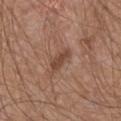workup: imaged on a skin check; not biopsied | automated metrics: a lesion color around L≈45 a*≈20 b*≈28 in CIELAB and about 9 CIELAB-L* units darker than the surrounding skin; a border-irregularity index near 3/10, internal color variation of about 1 on a 0–10 scale, and a peripheral color-asymmetry measure near 0.5 | subject: male, approximately 65 years of age | tile lighting: white-light illumination | image: ~15 mm tile from a whole-body skin photo | anatomic site: the front of the torso | size: ~3.5 mm (longest diameter).